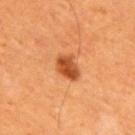- tile lighting: cross-polarized illumination
- subject: male, approximately 60 years of age
- diameter: ~3.5 mm (longest diameter)
- imaging modality: 15 mm crop, total-body photography
- location: the upper back